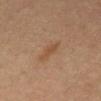Acquisition and patient details: A close-up tile cropped from a whole-body skin photograph, about 15 mm across. From the mid back. A male subject, approximately 65 years of age. Approximately 3.5 mm at its widest.